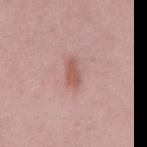The lesion-visualizer software estimated border irregularity of about 2 on a 0–10 scale, internal color variation of about 2 on a 0–10 scale, and a peripheral color-asymmetry measure near 1. It also reported a nevus-likeness score of about 40/100 and a detector confidence of about 100 out of 100 that the crop contains a lesion. Measured at roughly 3 mm in maximum diameter. The subject is a male in their 50s. Imaged with white-light lighting. A roughly 15 mm field-of-view crop from a total-body skin photograph. The lesion is located on the mid back.